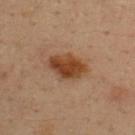The lesion was photographed on a routine skin check and not biopsied; there is no pathology result.
A 15 mm close-up tile from a total-body photography series done for melanoma screening.
Captured under cross-polarized illumination.
Located on the back.
The subject is a male approximately 35 years of age.